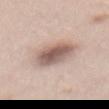<case>
<biopsy_status>not biopsied; imaged during a skin examination</biopsy_status>
<image>
  <source>total-body photography crop</source>
  <field_of_view_mm>15</field_of_view_mm>
</image>
<patient>
  <sex>female</sex>
  <age_approx>35</age_approx>
</patient>
<site>abdomen</site>
</case>